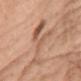Clinical impression: Imaged during a routine full-body skin examination; the lesion was not biopsied and no histopathology is available. Acquisition and patient details: Cropped from a whole-body photographic skin survey; the tile spans about 15 mm. An algorithmic analysis of the crop reported an eccentricity of roughly 0.8. And it measured a mean CIELAB color near L≈58 a*≈20 b*≈31, about 8 CIELAB-L* units darker than the surrounding skin, and a normalized border contrast of about 5.5. It also reported border irregularity of about 9.5 on a 0–10 scale, a within-lesion color-variation index near 8.5/10, and a peripheral color-asymmetry measure near 2.5. It also reported lesion-presence confidence of about 95/100. A female subject, in their 70s. The lesion is located on the chest. Measured at roughly 7.5 mm in maximum diameter.